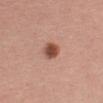Impression: Imaged during a routine full-body skin examination; the lesion was not biopsied and no histopathology is available. Acquisition and patient details: The lesion is on the left upper arm. Captured under white-light illumination. The lesion-visualizer software estimated an average lesion color of about L≈49 a*≈25 b*≈29 (CIELAB), a lesion–skin lightness drop of about 15, and a lesion-to-skin contrast of about 10.5 (normalized; higher = more distinct). The analysis additionally found a border-irregularity rating of about 2/10, a color-variation rating of about 3.5/10, and peripheral color asymmetry of about 1. A female subject, aged around 55. A lesion tile, about 15 mm wide, cut from a 3D total-body photograph.